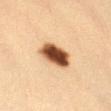Case summary:
– follow-up — imaged on a skin check; not biopsied
– size — ~4 mm (longest diameter)
– anatomic site — the left thigh
– image-analysis metrics — a lesion-to-skin contrast of about 15 (normalized; higher = more distinct); a within-lesion color-variation index near 7.5/10 and peripheral color asymmetry of about 2.5
– subject — female, aged 38–42
– acquisition — ~15 mm tile from a whole-body skin photo